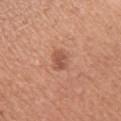biopsy status = imaged on a skin check; not biopsied
subject = female, approximately 30 years of age
lighting = white-light illumination
image source = total-body-photography crop, ~15 mm field of view
anatomic site = the right upper arm
diameter = ~2.5 mm (longest diameter)